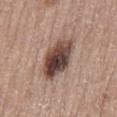Findings:
• workup: total-body-photography surveillance lesion; no biopsy
• tile lighting: white-light illumination
• image: 15 mm crop, total-body photography
• image-analysis metrics: a footprint of about 16 mm², an eccentricity of roughly 0.8, and a symmetry-axis asymmetry near 0.15; a mean CIELAB color near L≈44 a*≈18 b*≈23, about 18 CIELAB-L* units darker than the surrounding skin, and a normalized lesion–skin contrast near 12.5
• patient: male, aged 53–57